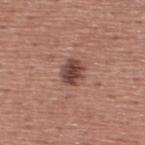Case summary:
* follow-up · catalogued during a skin exam; not biopsied
* location · the upper back
* subject · male, roughly 45 years of age
* imaging modality · 15 mm crop, total-body photography